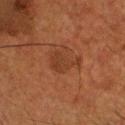Captured during whole-body skin photography for melanoma surveillance; the lesion was not biopsied. A 15 mm close-up extracted from a 3D total-body photography capture. The lesion-visualizer software estimated a nevus-likeness score of about 0/100 and lesion-presence confidence of about 100/100. Located on the head or neck. The patient is a male approximately 60 years of age. Imaged with cross-polarized lighting.